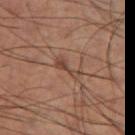  biopsy_status: not biopsied; imaged during a skin examination
  image:
    source: total-body photography crop
    field_of_view_mm: 15
  automated_metrics:
    area_mm2_approx: 4.0
    cielab_L: 42
    cielab_a: 18
    cielab_b: 26
    vs_skin_darker_L: 8.0
    vs_skin_contrast_norm: 6.5
    border_irregularity_0_10: 5.5
    peripheral_color_asymmetry: 0.5
  site: left thigh
  lesion_size:
    long_diameter_mm_approx: 3.5
  patient:
    sex: male
    age_approx: 60
  lighting: cross-polarized